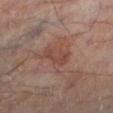Clinical impression: Part of a total-body skin-imaging series; this lesion was reviewed on a skin check and was not flagged for biopsy. Image and clinical context: An algorithmic analysis of the crop reported a lesion color around L≈41 a*≈20 b*≈24 in CIELAB, about 7 CIELAB-L* units darker than the surrounding skin, and a normalized lesion–skin contrast near 6. The lesion is located on the left lower leg. A 15 mm close-up tile from a total-body photography series done for melanoma screening. The recorded lesion diameter is about 3.5 mm. A male patient roughly 55 years of age.